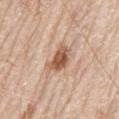Clinical impression:
Captured during whole-body skin photography for melanoma surveillance; the lesion was not biopsied.
Context:
Longest diameter approximately 3.5 mm. Cropped from a total-body skin-imaging series; the visible field is about 15 mm. An algorithmic analysis of the crop reported an outline eccentricity of about 0.75 (0 = round, 1 = elongated). The software also gave an average lesion color of about L≈57 a*≈20 b*≈32 (CIELAB), a lesion–skin lightness drop of about 14, and a normalized border contrast of about 9. And it measured a detector confidence of about 100 out of 100 that the crop contains a lesion. The lesion is located on the back. A male subject about 80 years old. The tile uses white-light illumination.